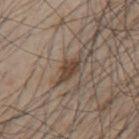<tbp_lesion>
<biopsy_status>not biopsied; imaged during a skin examination</biopsy_status>
<automated_metrics>
  <eccentricity>0.9</eccentricity>
  <shape_asymmetry>0.4</shape_asymmetry>
  <cielab_L>43</cielab_L>
  <cielab_a>14</cielab_a>
  <cielab_b>25</cielab_b>
  <vs_skin_darker_L>10.0</vs_skin_darker_L>
  <vs_skin_contrast_norm>8.5</vs_skin_contrast_norm>
  <border_irregularity_0_10>4.5</border_irregularity_0_10>
  <color_variation_0_10>2.0</color_variation_0_10>
  <peripheral_color_asymmetry>0.5</peripheral_color_asymmetry>
</automated_metrics>
<lighting>white-light</lighting>
<site>mid back</site>
<image>
  <source>total-body photography crop</source>
  <field_of_view_mm>15</field_of_view_mm>
</image>
<lesion_size>
  <long_diameter_mm_approx>4.5</long_diameter_mm_approx>
</lesion_size>
<patient>
  <sex>male</sex>
  <age_approx>45</age_approx>
</patient>
</tbp_lesion>